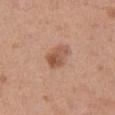Assessment:
Part of a total-body skin-imaging series; this lesion was reviewed on a skin check and was not flagged for biopsy.
Context:
On the left thigh. The tile uses white-light illumination. A female patient, aged 38 to 42. Measured at roughly 3.5 mm in maximum diameter. A roughly 15 mm field-of-view crop from a total-body skin photograph. The total-body-photography lesion software estimated an area of roughly 6 mm², an eccentricity of roughly 0.75, and a shape-asymmetry score of about 0.25 (0 = symmetric). The software also gave internal color variation of about 5 on a 0–10 scale and a peripheral color-asymmetry measure near 1.5.